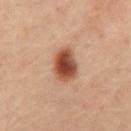The lesion was tiled from a total-body skin photograph and was not biopsied. Longest diameter approximately 4 mm. A male subject about 60 years old. Located on the abdomen. A 15 mm crop from a total-body photograph taken for skin-cancer surveillance.